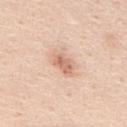Notes:
– biopsy status: imaged on a skin check; not biopsied
– illumination: white-light illumination
– size: about 3 mm
– image: 15 mm crop, total-body photography
– patient: male, in their mid-40s
– site: the mid back